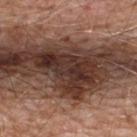Clinical impression:
No biopsy was performed on this lesion — it was imaged during a full skin examination and was not determined to be concerning.
Context:
A male patient aged approximately 80. This image is a 15 mm lesion crop taken from a total-body photograph. From the back. This is a white-light tile. The recorded lesion diameter is about 7 mm.